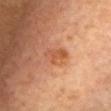notes: no biopsy performed (imaged during a skin exam); subject: female, aged 58 to 62; diameter: about 3.5 mm; site: the front of the torso; lighting: cross-polarized; imaging modality: 15 mm crop, total-body photography.